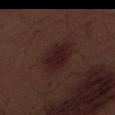Part of a total-body skin-imaging series; this lesion was reviewed on a skin check and was not flagged for biopsy. A region of skin cropped from a whole-body photographic capture, roughly 15 mm wide. The tile uses white-light illumination. The subject is a male aged 68 to 72. About 5 mm across. Automated image analysis of the tile measured a footprint of about 9.5 mm², an eccentricity of roughly 0.85, and a symmetry-axis asymmetry near 0.15. The analysis additionally found roughly 7 lightness units darker than nearby skin and a lesion-to-skin contrast of about 9 (normalized; higher = more distinct). The analysis additionally found a border-irregularity rating of about 2/10 and radial color variation of about 1. Located on the right thigh.